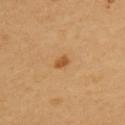biopsy status = total-body-photography surveillance lesion; no biopsy
subject = female, about 60 years old
image source = total-body-photography crop, ~15 mm field of view
illumination = cross-polarized illumination
site = the back
lesion size = ~2 mm (longest diameter)